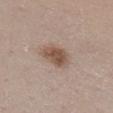Imaged during a routine full-body skin examination; the lesion was not biopsied and no histopathology is available.
From the chest.
Automated image analysis of the tile measured an average lesion color of about L≈52 a*≈17 b*≈26 (CIELAB) and a lesion–skin lightness drop of about 11. And it measured border irregularity of about 2 on a 0–10 scale, a color-variation rating of about 3/10, and peripheral color asymmetry of about 1.
A female patient, in their 50s.
A close-up tile cropped from a whole-body skin photograph, about 15 mm across.
Imaged with white-light lighting.
Longest diameter approximately 4 mm.